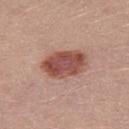notes: no biopsy performed (imaged during a skin exam) | lighting: white-light illumination | body site: the left thigh | subject: female, in their mid-20s | lesion size: ~5.5 mm (longest diameter) | acquisition: ~15 mm crop, total-body skin-cancer survey.